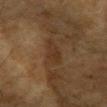<lesion>
<automated_metrics>
  <area_mm2_approx>4.5</area_mm2_approx>
  <shape_asymmetry>0.3</shape_asymmetry>
  <cielab_L>30</cielab_L>
  <cielab_a>15</cielab_a>
  <cielab_b>27</cielab_b>
  <vs_skin_darker_L>6.0</vs_skin_darker_L>
  <vs_skin_contrast_norm>6.0</vs_skin_contrast_norm>
  <border_irregularity_0_10>3.5</border_irregularity_0_10>
  <color_variation_0_10>1.5</color_variation_0_10>
  <peripheral_color_asymmetry>0.5</peripheral_color_asymmetry>
</automated_metrics>
<site>head or neck</site>
<lesion_size>
  <long_diameter_mm_approx>3.5</long_diameter_mm_approx>
</lesion_size>
<image>
  <source>total-body photography crop</source>
  <field_of_view_mm>15</field_of_view_mm>
</image>
<lighting>cross-polarized</lighting>
<patient>
  <sex>female</sex>
  <age_approx>60</age_approx>
</patient>
</lesion>